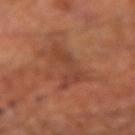Imaged during a routine full-body skin examination; the lesion was not biopsied and no histopathology is available.
The lesion is on the left forearm.
The subject is a male about 65 years old.
Approximately 6 mm at its widest.
A roughly 15 mm field-of-view crop from a total-body skin photograph.